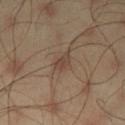| feature | finding |
|---|---|
| notes | total-body-photography surveillance lesion; no biopsy |
| acquisition | 15 mm crop, total-body photography |
| patient | male, aged 43 to 47 |
| illumination | cross-polarized illumination |
| diameter | ~2.5 mm (longest diameter) |
| site | the left thigh |